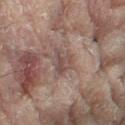Case summary:
* biopsy status — total-body-photography surveillance lesion; no biopsy
* lesion diameter — ~3 mm (longest diameter)
* patient — female, aged around 80
* tile lighting — cross-polarized illumination
* image — 15 mm crop, total-body photography
* body site — the right leg